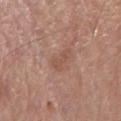Acquisition and patient details:
A 15 mm close-up tile from a total-body photography series done for melanoma screening. Captured under white-light illumination. A male patient, aged 63 to 67. On the chest.